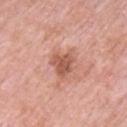Q: Is there a histopathology result?
A: no biopsy performed (imaged during a skin exam)
Q: What are the patient's age and sex?
A: male, aged 53–57
Q: What kind of image is this?
A: ~15 mm tile from a whole-body skin photo
Q: How was the tile lit?
A: white-light
Q: Lesion location?
A: the front of the torso
Q: Lesion size?
A: ≈4 mm
Q: Automated lesion metrics?
A: a lesion–skin lightness drop of about 11 and a lesion-to-skin contrast of about 7.5 (normalized; higher = more distinct)